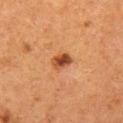| field | value |
|---|---|
| workup | no biopsy performed (imaged during a skin exam) |
| image source | 15 mm crop, total-body photography |
| patient | female, roughly 50 years of age |
| body site | the left thigh |